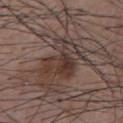Q: Is there a histopathology result?
A: catalogued during a skin exam; not biopsied